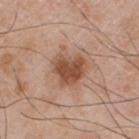Assessment: Captured during whole-body skin photography for melanoma surveillance; the lesion was not biopsied. Image and clinical context: A 15 mm close-up extracted from a 3D total-body photography capture. Imaged with white-light lighting. A male subject, aged 48–52. Longest diameter approximately 4 mm. From the chest. Automated tile analysis of the lesion measured an area of roughly 11 mm², an outline eccentricity of about 0.35 (0 = round, 1 = elongated), and a shape-asymmetry score of about 0.3 (0 = symmetric). The software also gave border irregularity of about 3 on a 0–10 scale, a within-lesion color-variation index near 3.5/10, and peripheral color asymmetry of about 1.